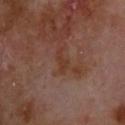biopsy status=imaged on a skin check; not biopsied
subject=male, approximately 70 years of age
anatomic site=the upper back
imaging modality=15 mm crop, total-body photography
illumination=cross-polarized